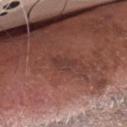Assessment: This lesion was catalogued during total-body skin photography and was not selected for biopsy. Acquisition and patient details: From the head or neck. A region of skin cropped from a whole-body photographic capture, roughly 15 mm wide. About 3 mm across. A male patient, about 75 years old. Automated image analysis of the tile measured a footprint of about 4 mm² and a symmetry-axis asymmetry near 0.45. The software also gave an average lesion color of about L≈37 a*≈21 b*≈23 (CIELAB), roughly 6 lightness units darker than nearby skin, and a lesion-to-skin contrast of about 5.5 (normalized; higher = more distinct). The analysis additionally found a color-variation rating of about 2/10. The analysis additionally found a nevus-likeness score of about 0/100.